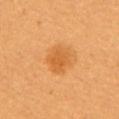Image and clinical context: About 3.5 mm across. A region of skin cropped from a whole-body photographic capture, roughly 15 mm wide. A female patient aged 28–32. An algorithmic analysis of the crop reported a classifier nevus-likeness of about 95/100 and a lesion-detection confidence of about 100/100. The lesion is located on the chest.